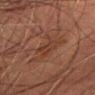workup = total-body-photography surveillance lesion; no biopsy
subject = male, aged 83 or older
diameter = ~3 mm (longest diameter)
image source = ~15 mm tile from a whole-body skin photo
illumination = cross-polarized
site = the abdomen